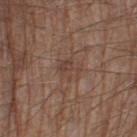Imaged during a routine full-body skin examination; the lesion was not biopsied and no histopathology is available. The subject is a male in their 80s. A close-up tile cropped from a whole-body skin photograph, about 15 mm across. Automated image analysis of the tile measured a lesion color around L≈42 a*≈17 b*≈25 in CIELAB and roughly 6 lightness units darker than nearby skin. The software also gave a border-irregularity rating of about 7/10, internal color variation of about 1.5 on a 0–10 scale, and peripheral color asymmetry of about 0.5. The software also gave a nevus-likeness score of about 0/100. The recorded lesion diameter is about 2.5 mm. From the left thigh. Captured under white-light illumination.